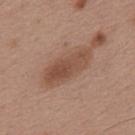Q: Was this lesion biopsied?
A: no biopsy performed (imaged during a skin exam)
Q: Illumination type?
A: white-light
Q: How was this image acquired?
A: 15 mm crop, total-body photography
Q: How large is the lesion?
A: ≈6.5 mm
Q: Where on the body is the lesion?
A: the upper back
Q: Patient demographics?
A: male, aged 53–57
Q: What did automated image analysis measure?
A: a mean CIELAB color near L≈49 a*≈20 b*≈28 and a lesion-to-skin contrast of about 7.5 (normalized; higher = more distinct); a border-irregularity index near 3/10 and internal color variation of about 4 on a 0–10 scale; a nevus-likeness score of about 0/100 and lesion-presence confidence of about 100/100